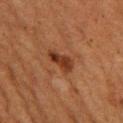Imaged during a routine full-body skin examination; the lesion was not biopsied and no histopathology is available. A region of skin cropped from a whole-body photographic capture, roughly 15 mm wide. A female patient approximately 50 years of age. The lesion is on the left thigh. The total-body-photography lesion software estimated a lesion area of about 5.5 mm² and an eccentricity of roughly 0.9. The analysis additionally found a border-irregularity rating of about 3.5/10, a within-lesion color-variation index near 3/10, and peripheral color asymmetry of about 1. And it measured a nevus-likeness score of about 75/100 and a detector confidence of about 100 out of 100 that the crop contains a lesion. This is a cross-polarized tile. About 3.5 mm across.